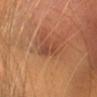follow-up: total-body-photography surveillance lesion; no biopsy
acquisition: ~15 mm crop, total-body skin-cancer survey
automated lesion analysis: a lesion area of about 5.5 mm², an outline eccentricity of about 0.65 (0 = round, 1 = elongated), and two-axis asymmetry of about 0.35; border irregularity of about 4.5 on a 0–10 scale, a within-lesion color-variation index near 0.5/10, and radial color variation of about 0; a classifier nevus-likeness of about 15/100 and a detector confidence of about 100 out of 100 that the crop contains a lesion
subject: female, about 30 years old
size: about 3 mm
site: the head or neck
illumination: cross-polarized illumination